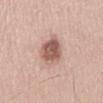Clinical impression:
The lesion was tiled from a total-body skin photograph and was not biopsied.
Image and clinical context:
Automated tile analysis of the lesion measured an area of roughly 10 mm² and two-axis asymmetry of about 0.2. The software also gave a lesion color around L≈57 a*≈20 b*≈25 in CIELAB, about 15 CIELAB-L* units darker than the surrounding skin, and a normalized lesion–skin contrast near 9.5. It also reported a classifier nevus-likeness of about 75/100 and lesion-presence confidence of about 100/100. About 5 mm across. The tile uses white-light illumination. A roughly 15 mm field-of-view crop from a total-body skin photograph. The subject is a male aged 43 to 47. The lesion is located on the mid back.